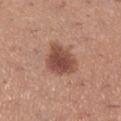This lesion was catalogued during total-body skin photography and was not selected for biopsy. The lesion is on the right lower leg. Cropped from a whole-body photographic skin survey; the tile spans about 15 mm. The subject is a female aged approximately 30.